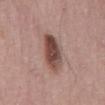biopsy_status: not biopsied; imaged during a skin examination
image:
  source: total-body photography crop
  field_of_view_mm: 15
lesion_size:
  long_diameter_mm_approx: 5.5
patient:
  sex: male
  age_approx: 55